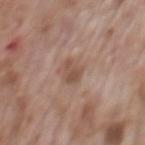Recorded during total-body skin imaging; not selected for excision or biopsy.
About 3 mm across.
A male patient, about 70 years old.
From the mid back.
A roughly 15 mm field-of-view crop from a total-body skin photograph.
The lesion-visualizer software estimated an area of roughly 4 mm² and a shape-asymmetry score of about 0.3 (0 = symmetric). The analysis additionally found a border-irregularity index near 3/10, a within-lesion color-variation index near 2/10, and radial color variation of about 0.5. It also reported a classifier nevus-likeness of about 0/100 and lesion-presence confidence of about 100/100.